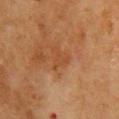Part of a total-body skin-imaging series; this lesion was reviewed on a skin check and was not flagged for biopsy.
Captured under cross-polarized illumination.
Approximately 3 mm at its widest.
The lesion is on the chest.
A 15 mm close-up tile from a total-body photography series done for melanoma screening.
A female subject, in their 50s.